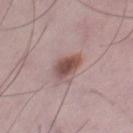| field | value |
|---|---|
| follow-up | imaged on a skin check; not biopsied |
| TBP lesion metrics | a lesion area of about 6.5 mm², an outline eccentricity of about 0.65 (0 = round, 1 = elongated), and two-axis asymmetry of about 0.25; an average lesion color of about L≈50 a*≈19 b*≈21 (CIELAB) and a lesion-to-skin contrast of about 9.5 (normalized; higher = more distinct) |
| lesion diameter | about 3.5 mm |
| subject | male, about 50 years old |
| tile lighting | white-light |
| imaging modality | ~15 mm crop, total-body skin-cancer survey |
| body site | the leg |